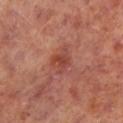Q: Is there a histopathology result?
A: no biopsy performed (imaged during a skin exam)
Q: Automated lesion metrics?
A: a footprint of about 6 mm², an eccentricity of roughly 0.3, and a shape-asymmetry score of about 0.3 (0 = symmetric); a lesion color around L≈44 a*≈28 b*≈28 in CIELAB, a lesion–skin lightness drop of about 8, and a normalized lesion–skin contrast near 6; a lesion-detection confidence of about 100/100
Q: Illumination type?
A: cross-polarized illumination
Q: What is the imaging modality?
A: 15 mm crop, total-body photography
Q: What is the lesion's diameter?
A: about 3 mm
Q: What are the patient's age and sex?
A: male, in their mid-60s
Q: Lesion location?
A: the left lower leg